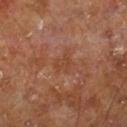Q: Was this lesion biopsied?
A: total-body-photography surveillance lesion; no biopsy
Q: What are the patient's age and sex?
A: male, aged 58–62
Q: Automated lesion metrics?
A: two-axis asymmetry of about 0.4; a lesion-to-skin contrast of about 5 (normalized; higher = more distinct); a border-irregularity index near 4.5/10, a within-lesion color-variation index near 1.5/10, and peripheral color asymmetry of about 0.5; a nevus-likeness score of about 0/100 and lesion-presence confidence of about 100/100
Q: Illumination type?
A: cross-polarized illumination
Q: Where on the body is the lesion?
A: the right leg
Q: What kind of image is this?
A: ~15 mm crop, total-body skin-cancer survey